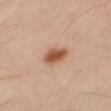Captured during whole-body skin photography for melanoma surveillance; the lesion was not biopsied. The lesion is located on the right thigh. A male patient, in their mid-50s. A close-up tile cropped from a whole-body skin photograph, about 15 mm across.